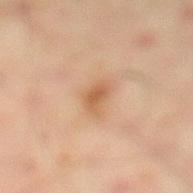Impression:
Part of a total-body skin-imaging series; this lesion was reviewed on a skin check and was not flagged for biopsy.
Context:
The lesion is on the left lower leg. A 15 mm close-up extracted from a 3D total-body photography capture. A male subject, approximately 45 years of age. Imaged with cross-polarized lighting. The total-body-photography lesion software estimated border irregularity of about 3 on a 0–10 scale and a within-lesion color-variation index near 2.5/10. The software also gave lesion-presence confidence of about 100/100.